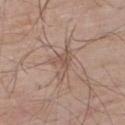biopsy status = catalogued during a skin exam; not biopsied
patient = male, in their mid- to late 60s
lesion diameter = about 4.5 mm
illumination = white-light illumination
TBP lesion metrics = a footprint of about 6 mm², a shape eccentricity near 0.9, and a symmetry-axis asymmetry near 0.55; a border-irregularity rating of about 7/10, a within-lesion color-variation index near 1.5/10, and peripheral color asymmetry of about 0.5; an automated nevus-likeness rating near 0 out of 100 and lesion-presence confidence of about 80/100
anatomic site = the upper back
image source = ~15 mm crop, total-body skin-cancer survey